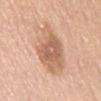No biopsy was performed on this lesion — it was imaged during a full skin examination and was not determined to be concerning. A female patient aged around 65. The lesion-visualizer software estimated an average lesion color of about L≈63 a*≈20 b*≈32 (CIELAB) and a normalized lesion–skin contrast near 7. It also reported a border-irregularity rating of about 2/10, a color-variation rating of about 4.5/10, and a peripheral color-asymmetry measure near 1.5. Longest diameter approximately 7.5 mm. Imaged with white-light lighting. The lesion is on the abdomen. A 15 mm close-up extracted from a 3D total-body photography capture.